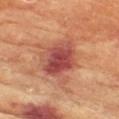notes: catalogued during a skin exam; not biopsied | illumination: cross-polarized illumination | diameter: ~5.5 mm (longest diameter) | subject: female, aged 78 to 82 | body site: the chest | acquisition: total-body-photography crop, ~15 mm field of view.